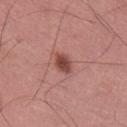{"biopsy_status": "not biopsied; imaged during a skin examination", "patient": {"sex": "male", "age_approx": 60}, "site": "leg", "image": {"source": "total-body photography crop", "field_of_view_mm": 15}, "lighting": "white-light", "automated_metrics": {"area_mm2_approx": 4.5, "eccentricity": 0.7, "lesion_detection_confidence_0_100": 100}, "lesion_size": {"long_diameter_mm_approx": 3.0}}